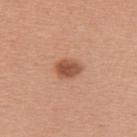Notes:
- follow-up: catalogued during a skin exam; not biopsied
- subject: female, aged 38 to 42
- image: 15 mm crop, total-body photography
- automated lesion analysis: a lesion area of about 6 mm², an outline eccentricity of about 0.7 (0 = round, 1 = elongated), and a shape-asymmetry score of about 0.15 (0 = symmetric); a border-irregularity index near 1.5/10, a color-variation rating of about 3/10, and peripheral color asymmetry of about 1; a nevus-likeness score of about 95/100 and a lesion-detection confidence of about 100/100
- illumination: white-light
- lesion size: ~3 mm (longest diameter)
- location: the upper back